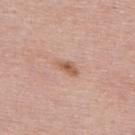Part of a total-body skin-imaging series; this lesion was reviewed on a skin check and was not flagged for biopsy.
Cropped from a whole-body photographic skin survey; the tile spans about 15 mm.
The patient is a male aged around 50.
From the upper back.